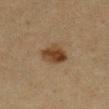Automated image analysis of the tile measured a shape-asymmetry score of about 0.25 (0 = symmetric). A female subject aged 53–57. From the leg. A 15 mm crop from a total-body photograph taken for skin-cancer surveillance. Approximately 4 mm at its widest. The tile uses cross-polarized illumination.